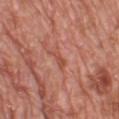Notes:
- follow-up — total-body-photography surveillance lesion; no biopsy
- imaging modality — ~15 mm crop, total-body skin-cancer survey
- subject — male, aged 78–82
- tile lighting — white-light illumination
- TBP lesion metrics — a footprint of about 2.5 mm², a shape eccentricity near 0.9, and a shape-asymmetry score of about 0.3 (0 = symmetric); a mean CIELAB color near L≈51 a*≈29 b*≈31; a lesion-detection confidence of about 90/100
- body site — the left lower leg